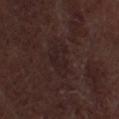Q: Was this lesion biopsied?
A: no biopsy performed (imaged during a skin exam)
Q: Who is the patient?
A: male, aged around 70
Q: Lesion location?
A: the right thigh
Q: What is the imaging modality?
A: total-body-photography crop, ~15 mm field of view
Q: How was the tile lit?
A: white-light
Q: What did automated image analysis measure?
A: an average lesion color of about L≈20 a*≈14 b*≈14 (CIELAB), roughly 4 lightness units darker than nearby skin, and a lesion-to-skin contrast of about 5.5 (normalized; higher = more distinct); a within-lesion color-variation index near 2.5/10 and peripheral color asymmetry of about 1; a nevus-likeness score of about 0/100 and a detector confidence of about 65 out of 100 that the crop contains a lesion
Q: How large is the lesion?
A: ≈4.5 mm